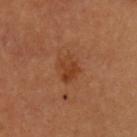Imaged during a routine full-body skin examination; the lesion was not biopsied and no histopathology is available. Located on the chest. Approximately 3 mm at its widest. Cropped from a whole-body photographic skin survey; the tile spans about 15 mm. The subject is a female about 50 years old. The tile uses cross-polarized illumination.